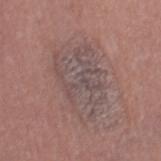<record>
<biopsy_status>not biopsied; imaged during a skin examination</biopsy_status>
<lighting>white-light</lighting>
<automated_metrics>
  <area_mm2_approx>27.0</area_mm2_approx>
  <eccentricity>0.8</eccentricity>
  <shape_asymmetry>0.3</shape_asymmetry>
  <border_irregularity_0_10>4.0</border_irregularity_0_10>
  <nevus_likeness_0_100>0</nevus_likeness_0_100>
  <lesion_detection_confidence_0_100>65</lesion_detection_confidence_0_100>
</automated_metrics>
<site>leg</site>
<image>
  <source>total-body photography crop</source>
  <field_of_view_mm>15</field_of_view_mm>
</image>
<patient>
  <sex>female</sex>
  <age_approx>60</age_approx>
</patient>
</record>